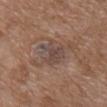<tbp_lesion>
  <biopsy_status>not biopsied; imaged during a skin examination</biopsy_status>
  <automated_metrics>
    <area_mm2_approx>7.5</area_mm2_approx>
    <eccentricity>0.6</eccentricity>
    <shape_asymmetry>0.45</shape_asymmetry>
    <cielab_L>44</cielab_L>
    <cielab_a>15</cielab_a>
    <cielab_b>21</cielab_b>
    <vs_skin_contrast_norm>6.5</vs_skin_contrast_norm>
    <border_irregularity_0_10>5.5</border_irregularity_0_10>
    <color_variation_0_10>2.5</color_variation_0_10>
    <peripheral_color_asymmetry>1.0</peripheral_color_asymmetry>
  </automated_metrics>
  <image>
    <source>total-body photography crop</source>
    <field_of_view_mm>15</field_of_view_mm>
  </image>
  <lighting>white-light</lighting>
  <site>back</site>
  <lesion_size>
    <long_diameter_mm_approx>4.0</long_diameter_mm_approx>
  </lesion_size>
  <patient>
    <sex>female</sex>
    <age_approx>70</age_approx>
  </patient>
</tbp_lesion>